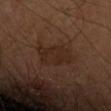| key | value |
|---|---|
| follow-up | total-body-photography surveillance lesion; no biopsy |
| patient | male, aged approximately 55 |
| site | the right forearm |
| imaging modality | total-body-photography crop, ~15 mm field of view |